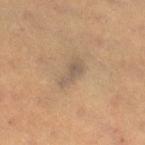Q: Was this lesion biopsied?
A: catalogued during a skin exam; not biopsied
Q: How was this image acquired?
A: ~15 mm tile from a whole-body skin photo
Q: Where on the body is the lesion?
A: the right thigh
Q: Patient demographics?
A: female, roughly 30 years of age